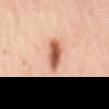| feature | finding |
|---|---|
| biopsy status | total-body-photography surveillance lesion; no biopsy |
| acquisition | total-body-photography crop, ~15 mm field of view |
| site | the mid back |
| subject | female, aged around 60 |
| automated lesion analysis | a lesion color around L≈58 a*≈25 b*≈34 in CIELAB and a normalized lesion–skin contrast near 11 |
| lesion size | ~4.5 mm (longest diameter) |
| lighting | cross-polarized |